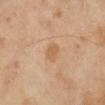Imaged during a routine full-body skin examination; the lesion was not biopsied and no histopathology is available.
On the right lower leg.
Automated image analysis of the tile measured a footprint of about 3.5 mm². It also reported an average lesion color of about L≈61 a*≈19 b*≈36 (CIELAB), about 7 CIELAB-L* units darker than the surrounding skin, and a normalized lesion–skin contrast near 5.5. The software also gave border irregularity of about 2.5 on a 0–10 scale and a within-lesion color-variation index near 1.5/10.
This image is a 15 mm lesion crop taken from a total-body photograph.
This is a cross-polarized tile.
About 2.5 mm across.
A female patient roughly 70 years of age.